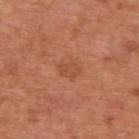- follow-up — no biopsy performed (imaged during a skin exam)
- image source — ~15 mm crop, total-body skin-cancer survey
- anatomic site — the upper back
- patient — male, in their mid- to late 60s
- TBP lesion metrics — a border-irregularity index near 2.5/10 and radial color variation of about 0.5; an automated nevus-likeness rating near 0 out of 100 and a detector confidence of about 100 out of 100 that the crop contains a lesion